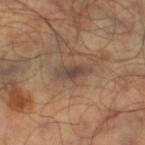Impression: Part of a total-body skin-imaging series; this lesion was reviewed on a skin check and was not flagged for biopsy. Acquisition and patient details: The recorded lesion diameter is about 4 mm. Automated image analysis of the tile measured a lesion area of about 6 mm² and a shape eccentricity near 0.9. The software also gave a lesion color around L≈44 a*≈15 b*≈24 in CIELAB, roughly 9 lightness units darker than nearby skin, and a normalized lesion–skin contrast near 8. It also reported peripheral color asymmetry of about 1.5. Cropped from a total-body skin-imaging series; the visible field is about 15 mm. On the right thigh. A male patient aged approximately 45.